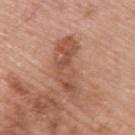patient: male, in their 80s
image: 15 mm crop, total-body photography
location: the upper back
illumination: white-light illumination
automated lesion analysis: a footprint of about 15 mm², an outline eccentricity of about 0.95 (0 = round, 1 = elongated), and a shape-asymmetry score of about 0.4 (0 = symmetric); an average lesion color of about L≈52 a*≈23 b*≈30 (CIELAB), about 10 CIELAB-L* units darker than the surrounding skin, and a normalized border contrast of about 7; a detector confidence of about 100 out of 100 that the crop contains a lesion